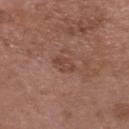The lesion was tiled from a total-body skin photograph and was not biopsied. Automated image analysis of the tile measured an outline eccentricity of about 0.8 (0 = round, 1 = elongated) and a shape-asymmetry score of about 0.25 (0 = symmetric). And it measured a border-irregularity index near 2.5/10, internal color variation of about 2.5 on a 0–10 scale, and a peripheral color-asymmetry measure near 1. And it measured a classifier nevus-likeness of about 5/100 and lesion-presence confidence of about 100/100. Cropped from a whole-body photographic skin survey; the tile spans about 15 mm. On the head or neck. A male subject, aged approximately 50.